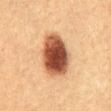biopsy status: total-body-photography surveillance lesion; no biopsy